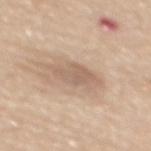workup: total-body-photography surveillance lesion; no biopsy
patient: female, roughly 60 years of age
site: the mid back
imaging modality: ~15 mm tile from a whole-body skin photo